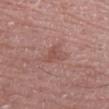Assessment:
Imaged during a routine full-body skin examination; the lesion was not biopsied and no histopathology is available.
Clinical summary:
From the right forearm. The subject is a female aged 63 to 67. The recorded lesion diameter is about 3 mm. This is a white-light tile. A 15 mm close-up tile from a total-body photography series done for melanoma screening.